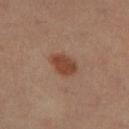A lesion tile, about 15 mm wide, cut from a 3D total-body photograph.
A female subject in their mid-30s.
Located on the right lower leg.